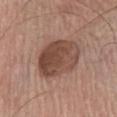Clinical impression: No biopsy was performed on this lesion — it was imaged during a full skin examination and was not determined to be concerning. Background: Longest diameter approximately 6 mm. A 15 mm close-up tile from a total-body photography series done for melanoma screening. A male patient, approximately 65 years of age. Imaged with white-light lighting. From the chest. Automated image analysis of the tile measured a lesion color around L≈46 a*≈19 b*≈26 in CIELAB and about 12 CIELAB-L* units darker than the surrounding skin. And it measured internal color variation of about 5.5 on a 0–10 scale and peripheral color asymmetry of about 2.